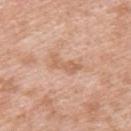Part of a total-body skin-imaging series; this lesion was reviewed on a skin check and was not flagged for biopsy.
Captured under white-light illumination.
The total-body-photography lesion software estimated a lesion area of about 4.5 mm², an eccentricity of roughly 0.9, and a shape-asymmetry score of about 0.4 (0 = symmetric). The analysis additionally found a mean CIELAB color near L≈62 a*≈21 b*≈33, roughly 8 lightness units darker than nearby skin, and a normalized lesion–skin contrast near 6. And it measured an automated nevus-likeness rating near 0 out of 100 and a detector confidence of about 100 out of 100 that the crop contains a lesion.
A male patient, about 40 years old.
A 15 mm close-up extracted from a 3D total-body photography capture.
From the upper back.
The recorded lesion diameter is about 3.5 mm.